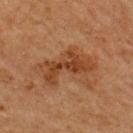Part of a total-body skin-imaging series; this lesion was reviewed on a skin check and was not flagged for biopsy. Located on the upper back. Captured under cross-polarized illumination. The total-body-photography lesion software estimated an average lesion color of about L≈36 a*≈21 b*≈32 (CIELAB) and a normalized lesion–skin contrast near 8. It also reported a nevus-likeness score of about 25/100. A roughly 15 mm field-of-view crop from a total-body skin photograph. The subject is a male approximately 65 years of age. Longest diameter approximately 6 mm.